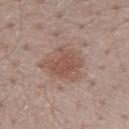The lesion was photographed on a routine skin check and not biopsied; there is no pathology result. From the mid back. A male patient, aged 53–57. A 15 mm close-up extracted from a 3D total-body photography capture. Captured under white-light illumination. The recorded lesion diameter is about 4.5 mm.